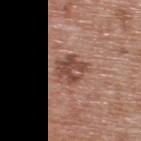Assessment: Captured during whole-body skin photography for melanoma surveillance; the lesion was not biopsied. Background: The lesion's longest dimension is about 4 mm. A 15 mm close-up extracted from a 3D total-body photography capture. Automated tile analysis of the lesion measured a footprint of about 8.5 mm² and an outline eccentricity of about 0.45 (0 = round, 1 = elongated). The analysis additionally found border irregularity of about 2.5 on a 0–10 scale, internal color variation of about 5 on a 0–10 scale, and radial color variation of about 2. And it measured an automated nevus-likeness rating near 15 out of 100. A male patient about 50 years old. The lesion is located on the upper back. The tile uses white-light illumination.